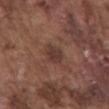The lesion was photographed on a routine skin check and not biopsied; there is no pathology result. A male patient in their mid-70s. Automated image analysis of the tile measured an outline eccentricity of about 0.45 (0 = round, 1 = elongated). It also reported an average lesion color of about L≈36 a*≈18 b*≈22 (CIELAB), a lesion–skin lightness drop of about 7, and a normalized lesion–skin contrast near 7. Located on the front of the torso. A region of skin cropped from a whole-body photographic capture, roughly 15 mm wide. This is a white-light tile.